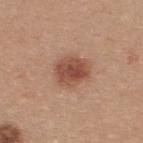Captured during whole-body skin photography for melanoma surveillance; the lesion was not biopsied. A lesion tile, about 15 mm wide, cut from a 3D total-body photograph. From the upper back. The subject is a male aged around 35. Imaged with white-light lighting. About 4 mm across.